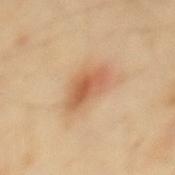Impression:
Recorded during total-body skin imaging; not selected for excision or biopsy.
Context:
The total-body-photography lesion software estimated a lesion area of about 10 mm² and a shape eccentricity near 0.85. And it measured a mean CIELAB color near L≈60 a*≈22 b*≈37, roughly 11 lightness units darker than nearby skin, and a lesion-to-skin contrast of about 7 (normalized; higher = more distinct). And it measured a nevus-likeness score of about 95/100 and lesion-presence confidence of about 100/100. The subject is a male aged approximately 40. Captured under cross-polarized illumination. A 15 mm close-up extracted from a 3D total-body photography capture. Approximately 5 mm at its widest. The lesion is on the mid back.